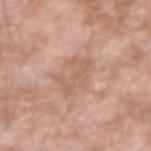Findings:
– lesion diameter: ~4 mm (longest diameter)
– acquisition: ~15 mm crop, total-body skin-cancer survey
– location: the right upper arm
– lighting: white-light
– subject: male, approximately 60 years of age
– image-analysis metrics: roughly 7 lightness units darker than nearby skin and a normalized border contrast of about 4.5; a nevus-likeness score of about 0/100 and lesion-presence confidence of about 100/100